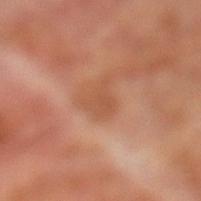Case summary:
• workup · imaged on a skin check; not biopsied
• lesion size · about 3.5 mm
• anatomic site · the left lower leg
• TBP lesion metrics · a lesion area of about 5.5 mm², a shape eccentricity near 0.7, and a shape-asymmetry score of about 0.45 (0 = symmetric); an automated nevus-likeness rating near 0 out of 100
• subject · male, approximately 70 years of age
• image · ~15 mm tile from a whole-body skin photo
• lighting · cross-polarized